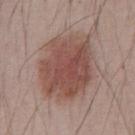Assessment: Imaged during a routine full-body skin examination; the lesion was not biopsied and no histopathology is available. Image and clinical context: The lesion is on the front of the torso. A 15 mm crop from a total-body photograph taken for skin-cancer surveillance. The recorded lesion diameter is about 7.5 mm. A male patient, about 40 years old. Automated image analysis of the tile measured a lesion area of about 35 mm² and a shape eccentricity near 0.6. The software also gave an average lesion color of about L≈49 a*≈20 b*≈24 (CIELAB), roughly 11 lightness units darker than nearby skin, and a normalized border contrast of about 8.5. The software also gave a border-irregularity index near 2/10, a color-variation rating of about 4.5/10, and peripheral color asymmetry of about 1.5. It also reported a lesion-detection confidence of about 100/100.